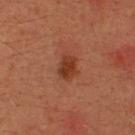biopsy status = catalogued during a skin exam; not biopsied | lesion diameter = about 3 mm | anatomic site = the upper back | image-analysis metrics = a symmetry-axis asymmetry near 0.25; an automated nevus-likeness rating near 85 out of 100 and lesion-presence confidence of about 100/100 | subject = male, in their mid- to late 30s | illumination = cross-polarized illumination | image source = 15 mm crop, total-body photography.